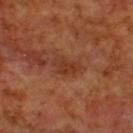Clinical impression: Imaged during a routine full-body skin examination; the lesion was not biopsied and no histopathology is available. Acquisition and patient details: A 15 mm crop from a total-body photograph taken for skin-cancer surveillance. Automated image analysis of the tile measured radial color variation of about 1. On the back. Imaged with cross-polarized lighting. A male patient about 70 years old. Measured at roughly 3.5 mm in maximum diameter.